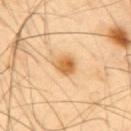• follow-up — no biopsy performed (imaged during a skin exam)
• image-analysis metrics — a mean CIELAB color near L≈66 a*≈22 b*≈45, about 13 CIELAB-L* units darker than the surrounding skin, and a lesion-to-skin contrast of about 9 (normalized; higher = more distinct)
• subject — male, aged approximately 40
• diameter — ≈3.5 mm
• anatomic site — the back
• imaging modality — total-body-photography crop, ~15 mm field of view